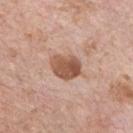Assessment: No biopsy was performed on this lesion — it was imaged during a full skin examination and was not determined to be concerning. Background: This is a white-light tile. About 3.5 mm across. A male subject aged 68 to 72. The lesion is located on the chest. Cropped from a whole-body photographic skin survey; the tile spans about 15 mm.